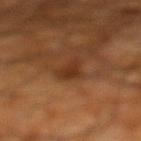A male patient, in their 60s. The lesion's longest dimension is about 3 mm. From the left lower leg. Captured under cross-polarized illumination. The total-body-photography lesion software estimated an area of roughly 4.5 mm², a shape eccentricity near 0.7, and two-axis asymmetry of about 0.45. And it measured internal color variation of about 3 on a 0–10 scale and peripheral color asymmetry of about 1. Cropped from a whole-body photographic skin survey; the tile spans about 15 mm.